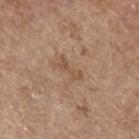No biopsy was performed on this lesion — it was imaged during a full skin examination and was not determined to be concerning.
Automated image analysis of the tile measured roughly 7 lightness units darker than nearby skin and a normalized border contrast of about 5.5. The software also gave border irregularity of about 6.5 on a 0–10 scale, a color-variation rating of about 0/10, and a peripheral color-asymmetry measure near 0.
The lesion's longest dimension is about 3 mm.
The lesion is on the left forearm.
A female patient, aged around 65.
This is a white-light tile.
Cropped from a whole-body photographic skin survey; the tile spans about 15 mm.